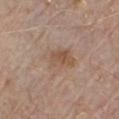Findings:
- site · the chest
- lighting · white-light
- image source · total-body-photography crop, ~15 mm field of view
- patient · male, aged 78–82